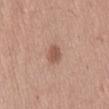Assessment: Recorded during total-body skin imaging; not selected for excision or biopsy. Context: The lesion is located on the abdomen. A lesion tile, about 15 mm wide, cut from a 3D total-body photograph. The subject is a male aged 53 to 57. About 2.5 mm across. The tile uses white-light illumination.